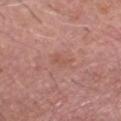No biopsy was performed on this lesion — it was imaged during a full skin examination and was not determined to be concerning. The recorded lesion diameter is about 2.5 mm. On the head or neck. A male patient, aged around 60. Cropped from a total-body skin-imaging series; the visible field is about 15 mm. Imaged with white-light lighting.